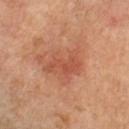<tbp_lesion>
<biopsy_status>not biopsied; imaged during a skin examination</biopsy_status>
<site>left lower leg</site>
<lesion_size>
  <long_diameter_mm_approx>5.0</long_diameter_mm_approx>
</lesion_size>
<patient>
  <age_approx>55</age_approx>
</patient>
<image>
  <source>total-body photography crop</source>
  <field_of_view_mm>15</field_of_view_mm>
</image>
</tbp_lesion>